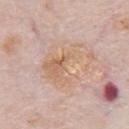Part of a total-body skin-imaging series; this lesion was reviewed on a skin check and was not flagged for biopsy. The patient is a male roughly 80 years of age. The recorded lesion diameter is about 6 mm. From the chest. The lesion-visualizer software estimated a lesion color around L≈65 a*≈18 b*≈31 in CIELAB, roughly 6 lightness units darker than nearby skin, and a normalized border contrast of about 6. A region of skin cropped from a whole-body photographic capture, roughly 15 mm wide. This is a white-light tile.